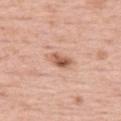<lesion>
<lighting>white-light</lighting>
<image>
  <source>total-body photography crop</source>
  <field_of_view_mm>15</field_of_view_mm>
</image>
<patient>
  <sex>female</sex>
  <age_approx>55</age_approx>
</patient>
<automated_metrics>
  <area_mm2_approx>4.0</area_mm2_approx>
  <eccentricity>0.8</eccentricity>
  <shape_asymmetry>0.3</shape_asymmetry>
  <vs_skin_darker_L>13.0</vs_skin_darker_L>
  <vs_skin_contrast_norm>8.5</vs_skin_contrast_norm>
  <border_irregularity_0_10>2.5</border_irregularity_0_10>
  <color_variation_0_10>5.5</color_variation_0_10>
  <nevus_likeness_0_100>80</nevus_likeness_0_100>
  <lesion_detection_confidence_0_100>100</lesion_detection_confidence_0_100>
</automated_metrics>
<site>leg</site>
</lesion>